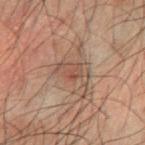<tbp_lesion>
  <biopsy_status>not biopsied; imaged during a skin examination</biopsy_status>
  <automated_metrics>
    <cielab_L>37</cielab_L>
    <cielab_a>15</cielab_a>
    <cielab_b>21</cielab_b>
    <vs_skin_darker_L>6.0</vs_skin_darker_L>
    <nevus_likeness_0_100>0</nevus_likeness_0_100>
    <lesion_detection_confidence_0_100>95</lesion_detection_confidence_0_100>
  </automated_metrics>
  <lesion_size>
    <long_diameter_mm_approx>4.0</long_diameter_mm_approx>
  </lesion_size>
  <site>right forearm</site>
  <image>
    <source>total-body photography crop</source>
    <field_of_view_mm>15</field_of_view_mm>
  </image>
  <lighting>cross-polarized</lighting>
  <patient>
    <sex>male</sex>
    <age_approx>50</age_approx>
  </patient>
</tbp_lesion>